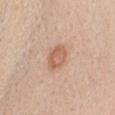Case summary:
- notes: total-body-photography surveillance lesion; no biopsy
- body site: the chest
- patient: female, in their mid-40s
- automated lesion analysis: border irregularity of about 1.5 on a 0–10 scale, internal color variation of about 3 on a 0–10 scale, and radial color variation of about 1
- lesion size: ≈3.5 mm
- acquisition: 15 mm crop, total-body photography
- lighting: white-light